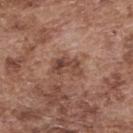The lesion was tiled from a total-body skin photograph and was not biopsied. A lesion tile, about 15 mm wide, cut from a 3D total-body photograph. Captured under white-light illumination. The subject is a male approximately 75 years of age. Approximately 3.5 mm at its widest. The lesion is on the upper back.